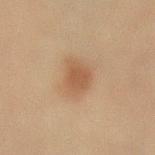The lesion was tiled from a total-body skin photograph and was not biopsied.
The subject is a male approximately 60 years of age.
From the lower back.
An algorithmic analysis of the crop reported a color-variation rating of about 1.5/10 and a peripheral color-asymmetry measure near 0.5. The analysis additionally found a classifier nevus-likeness of about 95/100.
The tile uses cross-polarized illumination.
Cropped from a total-body skin-imaging series; the visible field is about 15 mm.